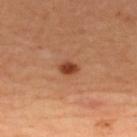The total-body-photography lesion software estimated a border-irregularity index near 1.5/10. The lesion is on the upper back. The tile uses cross-polarized illumination. A close-up tile cropped from a whole-body skin photograph, about 15 mm across. The lesion's longest dimension is about 2.5 mm. The subject is a female aged around 45.